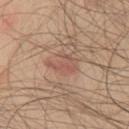notes: imaged on a skin check; not biopsied | location: the chest | lighting: white-light illumination | subject: male, roughly 45 years of age | diameter: ≈3.5 mm | image source: 15 mm crop, total-body photography.